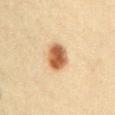<case>
<lighting>cross-polarized</lighting>
<patient>
  <sex>male</sex>
  <age_approx>40</age_approx>
</patient>
<site>left upper arm</site>
<automated_metrics>
  <cielab_L>53</cielab_L>
  <cielab_a>21</cielab_a>
  <cielab_b>35</cielab_b>
  <vs_skin_darker_L>17.0</vs_skin_darker_L>
  <vs_skin_contrast_norm>11.5</vs_skin_contrast_norm>
  <border_irregularity_0_10>1.0</border_irregularity_0_10>
  <color_variation_0_10>4.5</color_variation_0_10>
  <peripheral_color_asymmetry>1.5</peripheral_color_asymmetry>
</automated_metrics>
<lesion_size>
  <long_diameter_mm_approx>3.5</long_diameter_mm_approx>
</lesion_size>
<image>
  <source>total-body photography crop</source>
  <field_of_view_mm>15</field_of_view_mm>
</image>
</case>